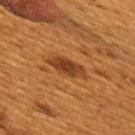Captured during whole-body skin photography for melanoma surveillance; the lesion was not biopsied. A 15 mm close-up extracted from a 3D total-body photography capture. On the upper back. Imaged with cross-polarized lighting. A female patient, in their 50s. Longest diameter approximately 4 mm.